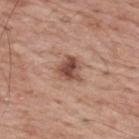Part of a total-body skin-imaging series; this lesion was reviewed on a skin check and was not flagged for biopsy. Captured under white-light illumination. The lesion is on the upper back. A male patient in their 70s. Measured at roughly 3 mm in maximum diameter. Cropped from a whole-body photographic skin survey; the tile spans about 15 mm.